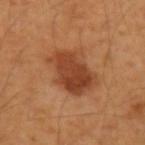The lesion was tiled from a total-body skin photograph and was not biopsied.